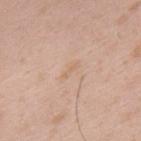Q: Was a biopsy performed?
A: catalogued during a skin exam; not biopsied